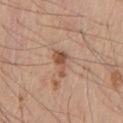{
  "image": {
    "source": "total-body photography crop",
    "field_of_view_mm": 15
  },
  "lighting": "white-light",
  "automated_metrics": {
    "area_mm2_approx": 4.5,
    "eccentricity": 0.8,
    "cielab_L": 53,
    "cielab_a": 21,
    "cielab_b": 31,
    "vs_skin_darker_L": 11.0,
    "vs_skin_contrast_norm": 7.5,
    "border_irregularity_0_10": 5.0,
    "color_variation_0_10": 4.0,
    "peripheral_color_asymmetry": 1.5
  },
  "patient": {
    "sex": "male",
    "age_approx": 60
  },
  "site": "front of the torso",
  "lesion_size": {
    "long_diameter_mm_approx": 3.0
  }
}